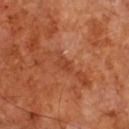Imaged during a routine full-body skin examination; the lesion was not biopsied and no histopathology is available. A 15 mm close-up tile from a total-body photography series done for melanoma screening. Longest diameter approximately 2.5 mm. The lesion is on the chest. The subject is a male about 60 years old.